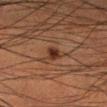Findings:
- follow-up · total-body-photography surveillance lesion; no biopsy
- diameter · ~1.5 mm (longest diameter)
- patient · male, aged 48 to 52
- body site · the right lower leg
- acquisition · ~15 mm crop, total-body skin-cancer survey
- TBP lesion metrics · a lesion area of about 2.5 mm² and two-axis asymmetry of about 0.2; a border-irregularity rating of about 1.5/10 and a within-lesion color-variation index near 1/10